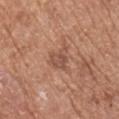Captured during whole-body skin photography for melanoma surveillance; the lesion was not biopsied.